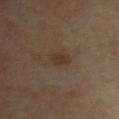{
  "biopsy_status": "not biopsied; imaged during a skin examination",
  "patient": {
    "sex": "female",
    "age_approx": 60
  },
  "site": "chest",
  "image": {
    "source": "total-body photography crop",
    "field_of_view_mm": 15
  }
}